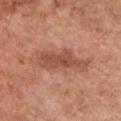Notes:
• notes — imaged on a skin check; not biopsied
• image source — total-body-photography crop, ~15 mm field of view
• diameter — ~6.5 mm (longest diameter)
• illumination — white-light illumination
• location — the chest
• patient — female, approximately 50 years of age